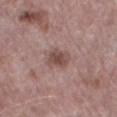The lesion is on the left thigh. A male subject, roughly 70 years of age. Cropped from a total-body skin-imaging series; the visible field is about 15 mm.